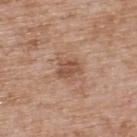Q: Was this lesion biopsied?
A: catalogued during a skin exam; not biopsied
Q: What lighting was used for the tile?
A: white-light illumination
Q: What is the anatomic site?
A: the back
Q: Lesion size?
A: ≈2.5 mm
Q: What are the patient's age and sex?
A: male, approximately 50 years of age
Q: What is the imaging modality?
A: ~15 mm tile from a whole-body skin photo
Q: Automated lesion metrics?
A: border irregularity of about 3 on a 0–10 scale and a color-variation rating of about 3/10